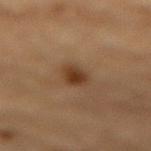The lesion was photographed on a routine skin check and not biopsied; there is no pathology result. The lesion's longest dimension is about 3.5 mm. The patient is a male in their mid- to late 80s or older. This image is a 15 mm lesion crop taken from a total-body photograph. An algorithmic analysis of the crop reported an outline eccentricity of about 0.75 (0 = round, 1 = elongated). And it measured roughly 9 lightness units darker than nearby skin and a lesion-to-skin contrast of about 8.5 (normalized; higher = more distinct). It also reported border irregularity of about 2.5 on a 0–10 scale, internal color variation of about 3 on a 0–10 scale, and radial color variation of about 1. The software also gave a classifier nevus-likeness of about 100/100 and a lesion-detection confidence of about 100/100. From the lower back. This is a cross-polarized tile.